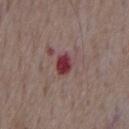Case summary:
• workup — no biopsy performed (imaged during a skin exam)
• size — ≈2.5 mm
• image — total-body-photography crop, ~15 mm field of view
• location — the chest
• illumination — white-light illumination
• TBP lesion metrics — an eccentricity of roughly 0.6 and two-axis asymmetry of about 0.15; an average lesion color of about L≈37 a*≈29 b*≈18 (CIELAB), roughly 14 lightness units darker than nearby skin, and a lesion-to-skin contrast of about 11.5 (normalized; higher = more distinct)
• subject — male, about 75 years old